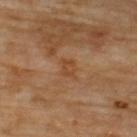An algorithmic analysis of the crop reported a symmetry-axis asymmetry near 0.35. And it measured a color-variation rating of about 2/10 and a peripheral color-asymmetry measure near 0.5. And it measured an automated nevus-likeness rating near 0 out of 100 and a lesion-detection confidence of about 100/100.
A male patient in their 70s.
On the upper back.
Captured under cross-polarized illumination.
Cropped from a total-body skin-imaging series; the visible field is about 15 mm.